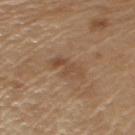{
  "biopsy_status": "not biopsied; imaged during a skin examination",
  "lesion_size": {
    "long_diameter_mm_approx": 4.5
  },
  "site": "left upper arm",
  "image": {
    "source": "total-body photography crop",
    "field_of_view_mm": 15
  },
  "patient": {
    "sex": "male",
    "age_approx": 70
  },
  "automated_metrics": {
    "cielab_L": 48,
    "cielab_a": 17,
    "cielab_b": 32,
    "vs_skin_darker_L": 7.0,
    "vs_skin_contrast_norm": 5.5,
    "nevus_likeness_0_100": 0,
    "lesion_detection_confidence_0_100": 100
  }
}